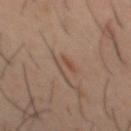| feature | finding |
|---|---|
| workup | total-body-photography surveillance lesion; no biopsy |
| lesion diameter | about 2.5 mm |
| lighting | cross-polarized illumination |
| acquisition | 15 mm crop, total-body photography |
| automated metrics | a lesion area of about 3.5 mm², an eccentricity of roughly 0.55, and a symmetry-axis asymmetry near 0.45; a lesion color around L≈47 a*≈17 b*≈27 in CIELAB, a lesion–skin lightness drop of about 6, and a normalized lesion–skin contrast near 5.5 |
| body site | the mid back |
| subject | male, aged 38 to 42 |